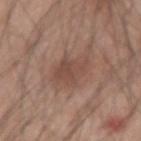workup = no biopsy performed (imaged during a skin exam)
lighting = white-light illumination
image = ~15 mm crop, total-body skin-cancer survey
diameter = ~4.5 mm (longest diameter)
site = the right forearm
subject = male, aged 43 to 47
automated lesion analysis = internal color variation of about 3 on a 0–10 scale and a peripheral color-asymmetry measure near 1